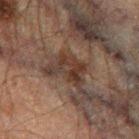No biopsy was performed on this lesion — it was imaged during a full skin examination and was not determined to be concerning. A 15 mm close-up extracted from a 3D total-body photography capture. The subject is a male about 60 years old. The tile uses cross-polarized illumination. Located on the left thigh.